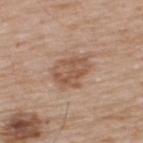Q: Is there a histopathology result?
A: catalogued during a skin exam; not biopsied
Q: How was this image acquired?
A: ~15 mm crop, total-body skin-cancer survey
Q: Patient demographics?
A: male, roughly 65 years of age
Q: What is the lesion's diameter?
A: about 4.5 mm
Q: How was the tile lit?
A: white-light illumination
Q: Automated lesion metrics?
A: border irregularity of about 2.5 on a 0–10 scale, internal color variation of about 4 on a 0–10 scale, and peripheral color asymmetry of about 1.5
Q: What is the anatomic site?
A: the upper back